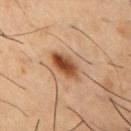Findings:
- biopsy status — imaged on a skin check; not biopsied